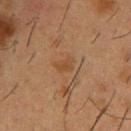  biopsy_status: not biopsied; imaged during a skin examination
  image:
    source: total-body photography crop
    field_of_view_mm: 15
  automated_metrics:
    area_mm2_approx: 3.5
    eccentricity: 0.8
    shape_asymmetry: 0.3
    nevus_likeness_0_100: 5
    lesion_detection_confidence_0_100: 100
  lighting: cross-polarized
  patient:
    sex: male
    age_approx: 55
  site: chest